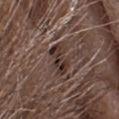| field | value |
|---|---|
| biopsy status | total-body-photography surveillance lesion; no biopsy |
| automated lesion analysis | a border-irregularity rating of about 4/10, a color-variation rating of about 6.5/10, and a peripheral color-asymmetry measure near 2.5 |
| subject | female, aged 68 to 72 |
| site | the head or neck |
| image | ~15 mm crop, total-body skin-cancer survey |